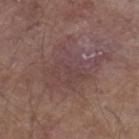Captured during whole-body skin photography for melanoma surveillance; the lesion was not biopsied. The lesion is on the right lower leg. A male patient, roughly 80 years of age. This is a white-light tile. A lesion tile, about 15 mm wide, cut from a 3D total-body photograph. Automated tile analysis of the lesion measured an area of roughly 27 mm², an outline eccentricity of about 0.6 (0 = round, 1 = elongated), and a shape-asymmetry score of about 0.4 (0 = symmetric). The software also gave a classifier nevus-likeness of about 0/100 and lesion-presence confidence of about 100/100. About 7.5 mm across.